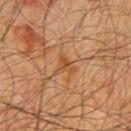follow-up: no biopsy performed (imaged during a skin exam); acquisition: 15 mm crop, total-body photography; subject: male, about 60 years old; location: the front of the torso; lesion diameter: about 3 mm.